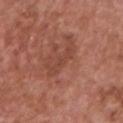Q: Was this lesion biopsied?
A: total-body-photography surveillance lesion; no biopsy
Q: How was this image acquired?
A: ~15 mm tile from a whole-body skin photo
Q: Lesion location?
A: the chest
Q: What are the patient's age and sex?
A: male, in their mid- to late 60s
Q: How large is the lesion?
A: ~4 mm (longest diameter)
Q: Illumination type?
A: white-light